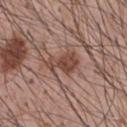Captured during whole-body skin photography for melanoma surveillance; the lesion was not biopsied.
Longest diameter approximately 4 mm.
A male subject, aged around 55.
The lesion is located on the front of the torso.
A 15 mm close-up extracted from a 3D total-body photography capture.
Captured under white-light illumination.
The total-body-photography lesion software estimated an average lesion color of about L≈46 a*≈20 b*≈25 (CIELAB), about 10 CIELAB-L* units darker than the surrounding skin, and a lesion-to-skin contrast of about 8 (normalized; higher = more distinct).